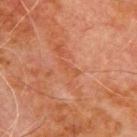Clinical impression:
The lesion was photographed on a routine skin check and not biopsied; there is no pathology result.
Context:
A male patient, aged 68–72. Located on the front of the torso. This image is a 15 mm lesion crop taken from a total-body photograph.